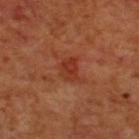The lesion was photographed on a routine skin check and not biopsied; there is no pathology result.
A male patient aged 68 to 72.
Located on the upper back.
A 15 mm crop from a total-body photograph taken for skin-cancer surveillance.
An algorithmic analysis of the crop reported a lesion color around L≈35 a*≈30 b*≈33 in CIELAB, roughly 7 lightness units darker than nearby skin, and a normalized lesion–skin contrast near 6.5. The software also gave a color-variation rating of about 2/10 and peripheral color asymmetry of about 0.5. The analysis additionally found a nevus-likeness score of about 5/100 and a lesion-detection confidence of about 100/100.
This is a cross-polarized tile.